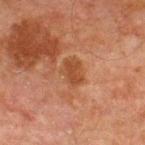biopsy status — no biopsy performed (imaged during a skin exam); patient — male, roughly 65 years of age; illumination — cross-polarized illumination; image source — total-body-photography crop, ~15 mm field of view; body site — the chest.